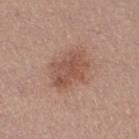Findings:
- automated metrics — a lesion color around L≈52 a*≈21 b*≈28 in CIELAB and roughly 9 lightness units darker than nearby skin; a within-lesion color-variation index near 3.5/10 and radial color variation of about 1; an automated nevus-likeness rating near 25 out of 100 and a lesion-detection confidence of about 100/100
- body site — the right thigh
- size — about 5 mm
- patient — female, roughly 20 years of age
- image source — 15 mm crop, total-body photography